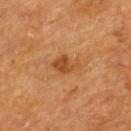Part of a total-body skin-imaging series; this lesion was reviewed on a skin check and was not flagged for biopsy. About 3.5 mm across. The lesion is located on the upper back. Imaged with cross-polarized lighting. A 15 mm close-up extracted from a 3D total-body photography capture. A female patient aged 53 to 57.